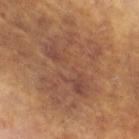No biopsy was performed on this lesion — it was imaged during a full skin examination and was not determined to be concerning. Cropped from a total-body skin-imaging series; the visible field is about 15 mm. The lesion's longest dimension is about 9 mm. The lesion is located on the left leg. The patient is a female aged approximately 65. This is a cross-polarized tile.